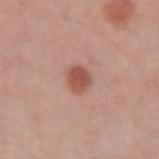Clinical impression: Captured during whole-body skin photography for melanoma surveillance; the lesion was not biopsied. Image and clinical context: The lesion is on the arm. Automated image analysis of the tile measured a footprint of about 5 mm², an eccentricity of roughly 0.7, and two-axis asymmetry of about 0.25. The analysis additionally found border irregularity of about 2.5 on a 0–10 scale, a color-variation rating of about 2/10, and a peripheral color-asymmetry measure near 0.5. It also reported a detector confidence of about 100 out of 100 that the crop contains a lesion. Cropped from a whole-body photographic skin survey; the tile spans about 15 mm. The patient is a male aged 58–62. Imaged with white-light lighting.